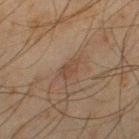Assessment: This lesion was catalogued during total-body skin photography and was not selected for biopsy. Context: A male patient about 45 years old. The lesion is located on the left thigh. A 15 mm close-up extracted from a 3D total-body photography capture. Captured under cross-polarized illumination. The recorded lesion diameter is about 3 mm. An algorithmic analysis of the crop reported a lesion–skin lightness drop of about 6. The software also gave lesion-presence confidence of about 100/100.